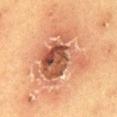Assessment: No biopsy was performed on this lesion — it was imaged during a full skin examination and was not determined to be concerning. Background: The lesion is located on the abdomen. A female subject about 50 years old. A region of skin cropped from a whole-body photographic capture, roughly 15 mm wide.